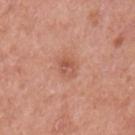patient = male, aged approximately 55; size = about 2.5 mm; anatomic site = the left upper arm; image source = ~15 mm tile from a whole-body skin photo; lighting = white-light illumination.